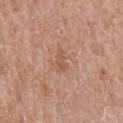A male patient aged around 65.
On the chest.
A roughly 15 mm field-of-view crop from a total-body skin photograph.
The lesion-visualizer software estimated a lesion color around L≈55 a*≈22 b*≈30 in CIELAB and roughly 7 lightness units darker than nearby skin. It also reported a border-irregularity rating of about 4.5/10, a color-variation rating of about 0/10, and a peripheral color-asymmetry measure near 0.
Approximately 3 mm at its widest.